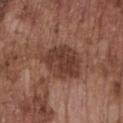The subject is a male aged around 75.
This image is a 15 mm lesion crop taken from a total-body photograph.
The recorded lesion diameter is about 6.5 mm.
The lesion is on the chest.
This is a white-light tile.